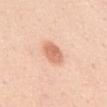<record>
<biopsy_status>not biopsied; imaged during a skin examination</biopsy_status>
</record>